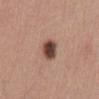Clinical impression:
No biopsy was performed on this lesion — it was imaged during a full skin examination and was not determined to be concerning.
Acquisition and patient details:
Automated image analysis of the tile measured a lesion area of about 7 mm² and an eccentricity of roughly 0.7. It also reported a lesion color around L≈45 a*≈20 b*≈25 in CIELAB and a normalized border contrast of about 12. And it measured internal color variation of about 6.5 on a 0–10 scale and a peripheral color-asymmetry measure near 2. It also reported a nevus-likeness score of about 95/100 and a detector confidence of about 100 out of 100 that the crop contains a lesion. The recorded lesion diameter is about 3.5 mm. Captured under white-light illumination. The lesion is located on the abdomen. A close-up tile cropped from a whole-body skin photograph, about 15 mm across. A male patient, about 55 years old.